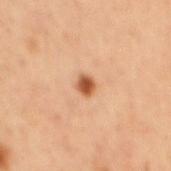follow-up — imaged on a skin check; not biopsied
subject — male, in their 60s
acquisition — ~15 mm crop, total-body skin-cancer survey
TBP lesion metrics — a border-irregularity rating of about 2/10 and a peripheral color-asymmetry measure near 1
tile lighting — cross-polarized
anatomic site — the mid back